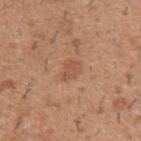  biopsy_status: not biopsied; imaged during a skin examination
  patient:
    sex: male
    age_approx: 40
  site: left upper arm
  lesion_size:
    long_diameter_mm_approx: 3.0
  image:
    source: total-body photography crop
    field_of_view_mm: 15